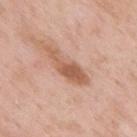Impression: Captured during whole-body skin photography for melanoma surveillance; the lesion was not biopsied. Image and clinical context: The patient is a male in their mid-50s. Imaged with white-light lighting. From the upper back. Cropped from a whole-body photographic skin survey; the tile spans about 15 mm.